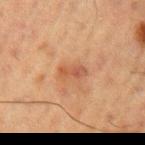Q: Is there a histopathology result?
A: catalogued during a skin exam; not biopsied
Q: What is the lesion's diameter?
A: about 3 mm
Q: Who is the patient?
A: male, approximately 60 years of age
Q: What is the anatomic site?
A: the left upper arm
Q: What is the imaging modality?
A: total-body-photography crop, ~15 mm field of view
Q: How was the tile lit?
A: cross-polarized
Q: Automated lesion metrics?
A: an average lesion color of about L≈42 a*≈20 b*≈29 (CIELAB) and about 7 CIELAB-L* units darker than the surrounding skin; a border-irregularity rating of about 2/10; an automated nevus-likeness rating near 5 out of 100 and lesion-presence confidence of about 100/100